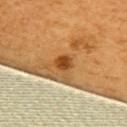Clinical impression: The lesion was tiled from a total-body skin photograph and was not biopsied. Background: On the upper back. A female subject aged 53–57. Cropped from a total-body skin-imaging series; the visible field is about 15 mm. Automated tile analysis of the lesion measured a footprint of about 4 mm², an eccentricity of roughly 0.5, and two-axis asymmetry of about 0.1. The software also gave a mean CIELAB color near L≈48 a*≈26 b*≈44 and a lesion–skin lightness drop of about 13.